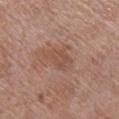{"biopsy_status": "not biopsied; imaged during a skin examination", "patient": {"sex": "female", "age_approx": 55}, "site": "right lower leg", "lesion_size": {"long_diameter_mm_approx": 4.0}, "lighting": "white-light", "image": {"source": "total-body photography crop", "field_of_view_mm": 15}}